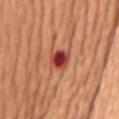<tbp_lesion>
<biopsy_status>not biopsied; imaged during a skin examination</biopsy_status>
</tbp_lesion>